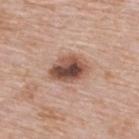Imaged during a routine full-body skin examination; the lesion was not biopsied and no histopathology is available. From the upper back. Imaged with white-light lighting. The patient is a male aged around 65. The recorded lesion diameter is about 4.5 mm. A 15 mm close-up extracted from a 3D total-body photography capture.